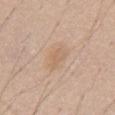Notes:
- notes · no biopsy performed (imaged during a skin exam)
- lesion size · ≈3.5 mm
- lighting · white-light
- subject · male, roughly 40 years of age
- image · ~15 mm tile from a whole-body skin photo
- anatomic site · the abdomen